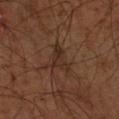| field | value |
|---|---|
| notes | total-body-photography surveillance lesion; no biopsy |
| lighting | cross-polarized |
| subject | male, aged around 45 |
| site | the left upper arm |
| automated metrics | border irregularity of about 6 on a 0–10 scale, a within-lesion color-variation index near 2.5/10, and radial color variation of about 1; an automated nevus-likeness rating near 0 out of 100 and a detector confidence of about 75 out of 100 that the crop contains a lesion |
| acquisition | total-body-photography crop, ~15 mm field of view |